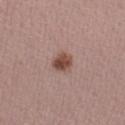Clinical impression:
The lesion was tiled from a total-body skin photograph and was not biopsied.
Image and clinical context:
The tile uses white-light illumination. An algorithmic analysis of the crop reported a lesion color around L≈47 a*≈20 b*≈25 in CIELAB, a lesion–skin lightness drop of about 13, and a lesion-to-skin contrast of about 9.5 (normalized; higher = more distinct). And it measured a classifier nevus-likeness of about 95/100 and a detector confidence of about 100 out of 100 that the crop contains a lesion. A close-up tile cropped from a whole-body skin photograph, about 15 mm across. The recorded lesion diameter is about 2.5 mm. The lesion is on the left forearm. A female patient, aged approximately 45.